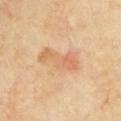Notes:
• biopsy status · imaged on a skin check; not biopsied
• subject · male, approximately 65 years of age
• site · the left upper arm
• image-analysis metrics · a shape eccentricity near 0.9 and a shape-asymmetry score of about 0.3 (0 = symmetric); roughly 8 lightness units darker than nearby skin and a normalized lesion–skin contrast near 5.5; a border-irregularity index near 4/10, a color-variation rating of about 7.5/10, and radial color variation of about 2.5
• lesion diameter · ≈5.5 mm
• image · ~15 mm crop, total-body skin-cancer survey